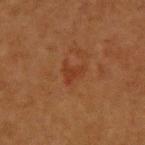Impression: Recorded during total-body skin imaging; not selected for excision or biopsy. Background: Located on the upper back. A female subject in their 40s. A 15 mm crop from a total-body photograph taken for skin-cancer surveillance. The recorded lesion diameter is about 3 mm. The total-body-photography lesion software estimated a mean CIELAB color near L≈33 a*≈23 b*≈30 and a normalized lesion–skin contrast near 5. It also reported border irregularity of about 5.5 on a 0–10 scale, a within-lesion color-variation index near 1.5/10, and a peripheral color-asymmetry measure near 0.5.